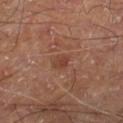follow-up = total-body-photography surveillance lesion; no biopsy | image = ~15 mm tile from a whole-body skin photo | subject = male, in their 70s | location = the right lower leg.